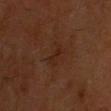No biopsy was performed on this lesion — it was imaged during a full skin examination and was not determined to be concerning. Measured at roughly 2.5 mm in maximum diameter. A male patient, in their 60s. A 15 mm crop from a total-body photograph taken for skin-cancer surveillance. The lesion is located on the head or neck. This is a cross-polarized tile. Automated image analysis of the tile measured a footprint of about 3 mm² and an eccentricity of roughly 0.85. It also reported a lesion color around L≈22 a*≈18 b*≈25 in CIELAB and a normalized border contrast of about 5. The analysis additionally found a classifier nevus-likeness of about 0/100 and lesion-presence confidence of about 100/100.